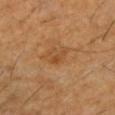Q: Was a biopsy performed?
A: total-body-photography surveillance lesion; no biopsy
Q: What is the imaging modality?
A: 15 mm crop, total-body photography
Q: Illumination type?
A: cross-polarized illumination
Q: What is the lesion's diameter?
A: about 2.5 mm
Q: What is the anatomic site?
A: the left lower leg
Q: Patient demographics?
A: male, roughly 60 years of age